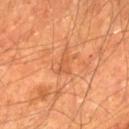Q: What kind of image is this?
A: ~15 mm tile from a whole-body skin photo
Q: Illumination type?
A: cross-polarized illumination
Q: Where on the body is the lesion?
A: the left lower leg
Q: Who is the patient?
A: male, roughly 65 years of age
Q: What is the lesion's diameter?
A: ~3 mm (longest diameter)